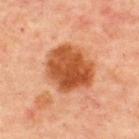Case summary:
- follow-up: no biopsy performed (imaged during a skin exam)
- imaging modality: ~15 mm tile from a whole-body skin photo
- diameter: ~6 mm (longest diameter)
- patient: male, aged 58 to 62
- anatomic site: the upper back